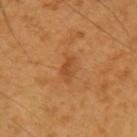<tbp_lesion>
<biopsy_status>not biopsied; imaged during a skin examination</biopsy_status>
<lesion_size>
  <long_diameter_mm_approx>2.5</long_diameter_mm_approx>
</lesion_size>
<site>right upper arm</site>
<patient>
  <sex>male</sex>
  <age_approx>60</age_approx>
</patient>
<image>
  <source>total-body photography crop</source>
  <field_of_view_mm>15</field_of_view_mm>
</image>
<lighting>cross-polarized</lighting>
</tbp_lesion>